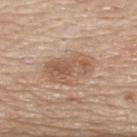acquisition: 15 mm crop, total-body photography | subject: male, aged approximately 80 | image-analysis metrics: an automated nevus-likeness rating near 50 out of 100 | diameter: ≈5.5 mm | illumination: white-light illumination | body site: the upper back.